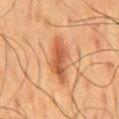On the back. The tile uses cross-polarized illumination. A male subject, aged around 60. Cropped from a whole-body photographic skin survey; the tile spans about 15 mm.